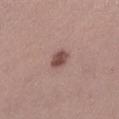Imaged during a routine full-body skin examination; the lesion was not biopsied and no histopathology is available.
The lesion is on the left lower leg.
A female patient, about 30 years old.
A roughly 15 mm field-of-view crop from a total-body skin photograph.